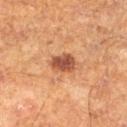biopsy status: catalogued during a skin exam; not biopsied | size: about 3 mm | automated metrics: a classifier nevus-likeness of about 90/100 and a detector confidence of about 100 out of 100 that the crop contains a lesion | patient: male, approximately 60 years of age | tile lighting: cross-polarized | location: the left lower leg | image source: 15 mm crop, total-body photography.